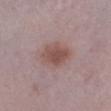{"biopsy_status": "not biopsied; imaged during a skin examination", "lighting": "white-light", "patient": {"sex": "male", "age_approx": 50}, "automated_metrics": {"eccentricity": 0.6, "cielab_L": 51, "cielab_a": 19, "cielab_b": 22, "vs_skin_darker_L": 9.0, "vs_skin_contrast_norm": 7.5, "border_irregularity_0_10": 1.5, "color_variation_0_10": 3.5, "peripheral_color_asymmetry": 1.0}, "site": "left lower leg", "image": {"source": "total-body photography crop", "field_of_view_mm": 15}, "lesion_size": {"long_diameter_mm_approx": 4.0}}